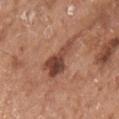This lesion was catalogued during total-body skin photography and was not selected for biopsy. A region of skin cropped from a whole-body photographic capture, roughly 15 mm wide. Imaged with white-light lighting. From the arm. The recorded lesion diameter is about 6.5 mm. A female subject, roughly 75 years of age.